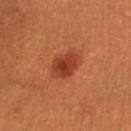Imaged during a routine full-body skin examination; the lesion was not biopsied and no histopathology is available. The lesion is on the left lower leg. A 15 mm close-up extracted from a 3D total-body photography capture. Captured under cross-polarized illumination. The recorded lesion diameter is about 4 mm. The patient is a female aged approximately 30.